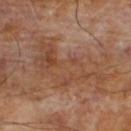follow-up = imaged on a skin check; not biopsied
patient = male, approximately 65 years of age
acquisition = ~15 mm tile from a whole-body skin photo
illumination = cross-polarized illumination
lesion diameter = ~9.5 mm (longest diameter)